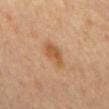Findings:
- tile lighting: cross-polarized
- body site: the abdomen
- patient: male, in their mid- to late 60s
- imaging modality: ~15 mm tile from a whole-body skin photo
- automated lesion analysis: a lesion color around L≈52 a*≈20 b*≈36 in CIELAB, about 9 CIELAB-L* units darker than the surrounding skin, and a lesion-to-skin contrast of about 7.5 (normalized; higher = more distinct); a nevus-likeness score of about 80/100 and a detector confidence of about 100 out of 100 that the crop contains a lesion